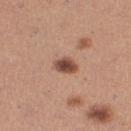No biopsy was performed on this lesion — it was imaged during a full skin examination and was not determined to be concerning.
This image is a 15 mm lesion crop taken from a total-body photograph.
The lesion is on the right thigh.
The patient is a female aged 28 to 32.
This is a white-light tile.
Measured at roughly 3 mm in maximum diameter.